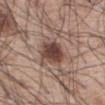This lesion was catalogued during total-body skin photography and was not selected for biopsy.
The patient is a male approximately 60 years of age.
Measured at roughly 3.5 mm in maximum diameter.
The lesion is on the abdomen.
A region of skin cropped from a whole-body photographic capture, roughly 15 mm wide.